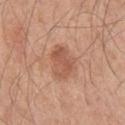Findings:
• follow-up — catalogued during a skin exam; not biopsied
• patient — male, in their mid- to late 40s
• location — the right upper arm
• illumination — white-light illumination
• image-analysis metrics — an area of roughly 9 mm², an eccentricity of roughly 0.65, and a symmetry-axis asymmetry near 0.25; a mean CIELAB color near L≈55 a*≈23 b*≈31 and a normalized border contrast of about 6.5; a border-irregularity rating of about 2.5/10, internal color variation of about 3.5 on a 0–10 scale, and radial color variation of about 1.5
• size — ≈4 mm
• image — total-body-photography crop, ~15 mm field of view